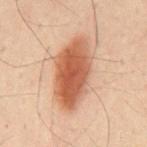Impression:
The lesion was photographed on a routine skin check and not biopsied; there is no pathology result.
Image and clinical context:
Automated tile analysis of the lesion measured an area of roughly 26 mm² and two-axis asymmetry of about 0.15. The analysis additionally found a lesion color around L≈52 a*≈23 b*≈32 in CIELAB, roughly 14 lightness units darker than nearby skin, and a normalized border contrast of about 10. It also reported border irregularity of about 2 on a 0–10 scale and a color-variation rating of about 5.5/10. The software also gave a lesion-detection confidence of about 100/100. Imaged with cross-polarized lighting. The lesion's longest dimension is about 8 mm. Cropped from a whole-body photographic skin survey; the tile spans about 15 mm. A male patient aged 48 to 52. On the mid back.